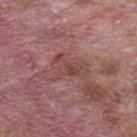This lesion was catalogued during total-body skin photography and was not selected for biopsy. Automated image analysis of the tile measured an area of roughly 7.5 mm², a shape eccentricity near 0.75, and a shape-asymmetry score of about 0.6 (0 = symmetric). The analysis additionally found an average lesion color of about L≈45 a*≈23 b*≈21 (CIELAB). It also reported a border-irregularity index near 8/10, a within-lesion color-variation index near 3/10, and radial color variation of about 1. And it measured an automated nevus-likeness rating near 0 out of 100 and a detector confidence of about 85 out of 100 that the crop contains a lesion. The recorded lesion diameter is about 4.5 mm. On the mid back. The patient is a male aged 68–72. A roughly 15 mm field-of-view crop from a total-body skin photograph. This is a white-light tile.